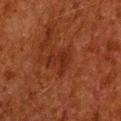{
  "biopsy_status": "not biopsied; imaged during a skin examination",
  "image": {
    "source": "total-body photography crop",
    "field_of_view_mm": 15
  },
  "lesion_size": {
    "long_diameter_mm_approx": 3.5
  },
  "patient": {
    "sex": "male",
    "age_approx": 80
  },
  "site": "head or neck",
  "automated_metrics": {
    "cielab_L": 23,
    "cielab_a": 23,
    "cielab_b": 27,
    "vs_skin_darker_L": 5.0,
    "vs_skin_contrast_norm": 5.5,
    "nevus_likeness_0_100": 0,
    "lesion_detection_confidence_0_100": 100
  }
}